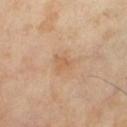Q: Was a biopsy performed?
A: imaged on a skin check; not biopsied
Q: What did automated image analysis measure?
A: a footprint of about 4.5 mm² and a shape eccentricity near 0.6; about 6 CIELAB-L* units darker than the surrounding skin and a lesion-to-skin contrast of about 4.5 (normalized; higher = more distinct); a lesion-detection confidence of about 100/100
Q: Who is the patient?
A: female, aged 68–72
Q: Where on the body is the lesion?
A: the leg
Q: Lesion size?
A: ~2.5 mm (longest diameter)
Q: What lighting was used for the tile?
A: cross-polarized illumination
Q: What is the imaging modality?
A: ~15 mm tile from a whole-body skin photo